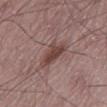Q: Was this lesion biopsied?
A: imaged on a skin check; not biopsied
Q: What are the patient's age and sex?
A: male, in their mid-60s
Q: Lesion size?
A: ≈4 mm
Q: Lesion location?
A: the left thigh
Q: Illumination type?
A: white-light
Q: What kind of image is this?
A: total-body-photography crop, ~15 mm field of view